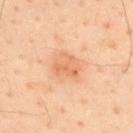notes = imaged on a skin check; not biopsied | acquisition = 15 mm crop, total-body photography | patient = male, about 45 years old | tile lighting = cross-polarized | location = the back.